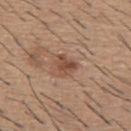Cropped from a total-body skin-imaging series; the visible field is about 15 mm. Longest diameter approximately 2.5 mm. The tile uses white-light illumination. On the upper back. The total-body-photography lesion software estimated a mean CIELAB color near L≈48 a*≈20 b*≈29, about 10 CIELAB-L* units darker than the surrounding skin, and a normalized lesion–skin contrast near 7.5. And it measured lesion-presence confidence of about 100/100. The subject is a male about 60 years old.